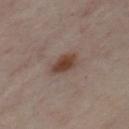Assessment:
Imaged during a routine full-body skin examination; the lesion was not biopsied and no histopathology is available.
Image and clinical context:
A 15 mm crop from a total-body photograph taken for skin-cancer surveillance. The tile uses cross-polarized illumination. The recorded lesion diameter is about 3 mm. The patient is roughly 55 years of age. The lesion is on the front of the torso.